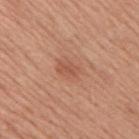Clinical impression:
The lesion was tiled from a total-body skin photograph and was not biopsied.
Acquisition and patient details:
A male patient, approximately 55 years of age. Cropped from a total-body skin-imaging series; the visible field is about 15 mm. Located on the right upper arm.